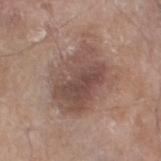No biopsy was performed on this lesion — it was imaged during a full skin examination and was not determined to be concerning.
This is a white-light tile.
A roughly 15 mm field-of-view crop from a total-body skin photograph.
The lesion is located on the left thigh.
A male patient approximately 80 years of age.